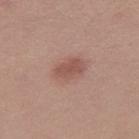| field | value |
|---|---|
| notes | catalogued during a skin exam; not biopsied |
| location | the leg |
| automated metrics | a footprint of about 6.5 mm², a shape eccentricity near 0.8, and a shape-asymmetry score of about 0.25 (0 = symmetric); a lesion color around L≈52 a*≈22 b*≈25 in CIELAB, roughly 9 lightness units darker than nearby skin, and a lesion-to-skin contrast of about 6.5 (normalized; higher = more distinct); a detector confidence of about 100 out of 100 that the crop contains a lesion |
| illumination | white-light illumination |
| image | 15 mm crop, total-body photography |
| lesion diameter | ~3.5 mm (longest diameter) |
| patient | female, approximately 20 years of age |